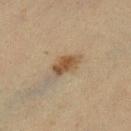Captured during whole-body skin photography for melanoma surveillance; the lesion was not biopsied.
The tile uses cross-polarized illumination.
The recorded lesion diameter is about 3.5 mm.
Automated image analysis of the tile measured a lesion area of about 6 mm², a shape eccentricity near 0.85, and a symmetry-axis asymmetry near 0.25. The software also gave a border-irregularity rating of about 2.5/10 and a within-lesion color-variation index near 3/10. And it measured a nevus-likeness score of about 85/100 and a detector confidence of about 100 out of 100 that the crop contains a lesion.
A female subject, aged around 55.
A 15 mm close-up extracted from a 3D total-body photography capture.
The lesion is on the left lower leg.